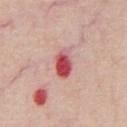<tbp_lesion>
  <biopsy_status>not biopsied; imaged during a skin examination</biopsy_status>
  <patient>
    <sex>male</sex>
    <age_approx>60</age_approx>
  </patient>
  <lighting>white-light</lighting>
  <image>
    <source>total-body photography crop</source>
    <field_of_view_mm>15</field_of_view_mm>
  </image>
  <lesion_size>
    <long_diameter_mm_approx>3.5</long_diameter_mm_approx>
  </lesion_size>
  <site>chest</site>
</tbp_lesion>